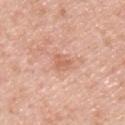The lesion was photographed on a routine skin check and not biopsied; there is no pathology result. The lesion is located on the upper back. The subject is a male in their mid-40s. A lesion tile, about 15 mm wide, cut from a 3D total-body photograph.